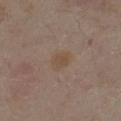Impression:
The lesion was tiled from a total-body skin photograph and was not biopsied.
Clinical summary:
A female subject, approximately 35 years of age. A close-up tile cropped from a whole-body skin photograph, about 15 mm across. Automated tile analysis of the lesion measured a mean CIELAB color near L≈45 a*≈13 b*≈25 and a normalized lesion–skin contrast near 5.5. The analysis additionally found a border-irregularity index near 1.5/10 and a peripheral color-asymmetry measure near 0.5. The lesion is located on the right lower leg.